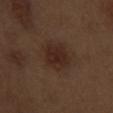image:
  source: total-body photography crop
  field_of_view_mm: 15
automated_metrics:
  cielab_L: 26
  cielab_a: 17
  cielab_b: 22
  vs_skin_contrast_norm: 7.5
  border_irregularity_0_10: 2.5
  color_variation_0_10: 2.5
  peripheral_color_asymmetry: 1.0
patient:
  sex: male
  age_approx: 70
site: left upper arm
lighting: white-light